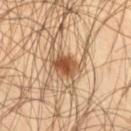Clinical summary: On the right thigh. The tile uses cross-polarized illumination. About 4 mm across. The subject is a male in their mid-40s. Automated image analysis of the tile measured a lesion area of about 7.5 mm², a shape eccentricity near 0.7, and a symmetry-axis asymmetry near 0.25. It also reported roughly 13 lightness units darker than nearby skin. The software also gave an automated nevus-likeness rating near 95 out of 100 and a lesion-detection confidence of about 100/100. A roughly 15 mm field-of-view crop from a total-body skin photograph.